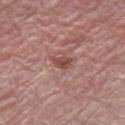| feature | finding |
|---|---|
| notes | imaged on a skin check; not biopsied |
| subject | female, aged approximately 55 |
| diameter | about 3 mm |
| image-analysis metrics | a mean CIELAB color near L≈48 a*≈22 b*≈24 and a normalized lesion–skin contrast near 7.5; border irregularity of about 3.5 on a 0–10 scale, a within-lesion color-variation index near 2.5/10, and radial color variation of about 1; a nevus-likeness score of about 0/100 and lesion-presence confidence of about 100/100 |
| image | 15 mm crop, total-body photography |
| tile lighting | white-light |
| anatomic site | the right thigh |